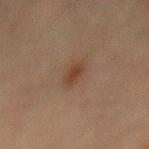workup=imaged on a skin check; not biopsied
location=the abdomen
size=≈2.5 mm
subject=male, roughly 70 years of age
image source=~15 mm crop, total-body skin-cancer survey
tile lighting=cross-polarized